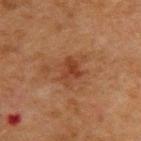Assessment: Recorded during total-body skin imaging; not selected for excision or biopsy. Acquisition and patient details: A male subject aged approximately 50. Longest diameter approximately 3 mm. On the upper back. Automated image analysis of the tile measured an area of roughly 5 mm², an eccentricity of roughly 0.65, and a symmetry-axis asymmetry near 0.3. The software also gave an automated nevus-likeness rating near 5 out of 100 and a lesion-detection confidence of about 100/100. The tile uses cross-polarized illumination. A 15 mm close-up extracted from a 3D total-body photography capture.